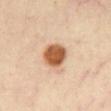Impression: No biopsy was performed on this lesion — it was imaged during a full skin examination and was not determined to be concerning. Background: A roughly 15 mm field-of-view crop from a total-body skin photograph. An algorithmic analysis of the crop reported a color-variation rating of about 3/10 and peripheral color asymmetry of about 1. The analysis additionally found a nevus-likeness score of about 100/100 and a detector confidence of about 100 out of 100 that the crop contains a lesion. From the chest. The patient is a female aged approximately 35. Measured at roughly 3.5 mm in maximum diameter. Imaged with cross-polarized lighting.